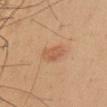notes = catalogued during a skin exam; not biopsied | lesion diameter = ~2.5 mm (longest diameter) | imaging modality = total-body-photography crop, ~15 mm field of view | body site = the chest | automated lesion analysis = a mean CIELAB color near L≈57 a*≈22 b*≈35 and a lesion–skin lightness drop of about 8; a within-lesion color-variation index near 2/10 and peripheral color asymmetry of about 0.5 | subject = male, roughly 55 years of age.